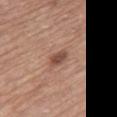workup: imaged on a skin check; not biopsied | image: total-body-photography crop, ~15 mm field of view | lighting: white-light | body site: the leg | subject: female, aged around 75.